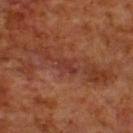Findings:
– lesion diameter: ~2.5 mm (longest diameter)
– image source: ~15 mm tile from a whole-body skin photo
– site: the upper back
– patient: male, roughly 70 years of age